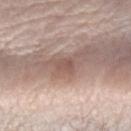biopsy status=no biopsy performed (imaged during a skin exam); subject=female, aged 53–57; image source=total-body-photography crop, ~15 mm field of view; size=≈3 mm; anatomic site=the right forearm; tile lighting=white-light.